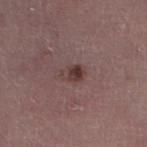Notes:
* notes: no biopsy performed (imaged during a skin exam)
* image source: ~15 mm tile from a whole-body skin photo
* site: the leg
* size: about 2.5 mm
* subject: female, aged around 45
* automated lesion analysis: an outline eccentricity of about 0.7 (0 = round, 1 = elongated); a lesion color around L≈37 a*≈18 b*≈18 in CIELAB, roughly 9 lightness units darker than nearby skin, and a lesion-to-skin contrast of about 8 (normalized; higher = more distinct); a classifier nevus-likeness of about 90/100 and a detector confidence of about 100 out of 100 that the crop contains a lesion